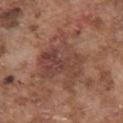No biopsy was performed on this lesion — it was imaged during a full skin examination and was not determined to be concerning.
Approximately 6.5 mm at its widest.
Automated image analysis of the tile measured a lesion color around L≈43 a*≈21 b*≈25 in CIELAB and a normalized lesion–skin contrast near 7.
The lesion is located on the chest.
This image is a 15 mm lesion crop taken from a total-body photograph.
This is a white-light tile.
The patient is a male aged approximately 75.